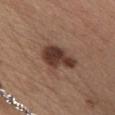Clinical impression:
No biopsy was performed on this lesion — it was imaged during a full skin examination and was not determined to be concerning.
Clinical summary:
The lesion is on the front of the torso. Longest diameter approximately 5 mm. Captured under white-light illumination. The lesion-visualizer software estimated a mean CIELAB color near L≈37 a*≈19 b*≈24, a lesion–skin lightness drop of about 14, and a lesion-to-skin contrast of about 11.5 (normalized; higher = more distinct). It also reported radial color variation of about 1.5. Cropped from a total-body skin-imaging series; the visible field is about 15 mm. The patient is a female in their mid- to late 40s.